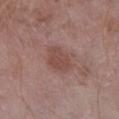workup = imaged on a skin check; not biopsied
illumination = white-light illumination
subject = male, in their mid- to late 50s
site = the right lower leg
size = ≈3.5 mm
imaging modality = 15 mm crop, total-body photography